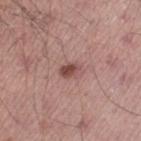Findings:
* image-analysis metrics · a lesion area of about 4 mm² and two-axis asymmetry of about 0.2
* anatomic site · the left thigh
* lesion diameter · ~2.5 mm (longest diameter)
* image · total-body-photography crop, ~15 mm field of view
* lighting · white-light
* patient · male, approximately 40 years of age